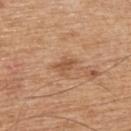biopsy status = catalogued during a skin exam; not biopsied | diameter = about 2.5 mm | site = the upper back | image source = total-body-photography crop, ~15 mm field of view | patient = male, aged 63–67.